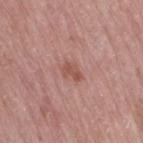Clinical impression:
The lesion was photographed on a routine skin check and not biopsied; there is no pathology result.
Acquisition and patient details:
The lesion is on the right thigh. A female subject aged 63–67. Measured at roughly 2.5 mm in maximum diameter. The tile uses white-light illumination. A 15 mm crop from a total-body photograph taken for skin-cancer surveillance.